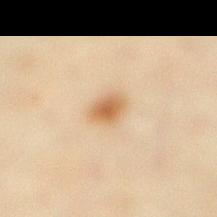biopsy status=no biopsy performed (imaged during a skin exam)
lesion size=~3 mm (longest diameter)
acquisition=total-body-photography crop, ~15 mm field of view
automated lesion analysis=an average lesion color of about L≈56 a*≈16 b*≈34 (CIELAB), roughly 10 lightness units darker than nearby skin, and a normalized border contrast of about 8
subject=female, roughly 65 years of age
lighting=cross-polarized
location=the leg